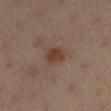{
  "biopsy_status": "not biopsied; imaged during a skin examination",
  "lighting": "cross-polarized",
  "automated_metrics": {
    "area_mm2_approx": 5.0,
    "eccentricity": 0.6,
    "shape_asymmetry": 0.25,
    "border_irregularity_0_10": 2.0,
    "color_variation_0_10": 2.5,
    "peripheral_color_asymmetry": 1.0,
    "nevus_likeness_0_100": 90,
    "lesion_detection_confidence_0_100": 100
  },
  "patient": {
    "sex": "female",
    "age_approx": 35
  },
  "lesion_size": {
    "long_diameter_mm_approx": 2.5
  },
  "image": {
    "source": "total-body photography crop",
    "field_of_view_mm": 15
  },
  "site": "left lower leg"
}